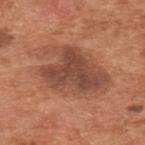Case summary:
– biopsy status: no biopsy performed (imaged during a skin exam)
– illumination: white-light
– imaging modality: 15 mm crop, total-body photography
– TBP lesion metrics: an area of roughly 33 mm², an outline eccentricity of about 0.75 (0 = round, 1 = elongated), and a shape-asymmetry score of about 0.2 (0 = symmetric); a mean CIELAB color near L≈48 a*≈24 b*≈30, about 10 CIELAB-L* units darker than the surrounding skin, and a normalized border contrast of about 8; a border-irregularity index near 4/10 and radial color variation of about 1.5; a nevus-likeness score of about 60/100
– anatomic site: the upper back
– patient: male, approximately 65 years of age